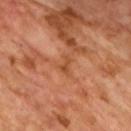This lesion was catalogued during total-body skin photography and was not selected for biopsy.
A male subject, aged 63–67.
This image is a 15 mm lesion crop taken from a total-body photograph.
Automated tile analysis of the lesion measured a lesion area of about 2.5 mm² and an eccentricity of roughly 0.8. It also reported a classifier nevus-likeness of about 0/100.
Imaged with cross-polarized lighting.